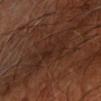notes = catalogued during a skin exam; not biopsied
anatomic site = the left forearm
tile lighting = cross-polarized illumination
lesion diameter = ~6 mm (longest diameter)
subject = approximately 65 years of age
image = ~15 mm tile from a whole-body skin photo